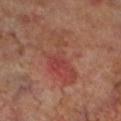No biopsy was performed on this lesion — it was imaged during a full skin examination and was not determined to be concerning.
A lesion tile, about 15 mm wide, cut from a 3D total-body photograph.
Automated image analysis of the tile measured an average lesion color of about L≈42 a*≈27 b*≈26 (CIELAB), roughly 7 lightness units darker than nearby skin, and a normalized border contrast of about 5.5. The software also gave border irregularity of about 9 on a 0–10 scale, a color-variation rating of about 3/10, and peripheral color asymmetry of about 1.
This is a cross-polarized tile.
The subject is a male in their 70s.
The lesion is located on the left lower leg.
The recorded lesion diameter is about 7 mm.